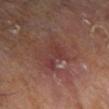follow-up — catalogued during a skin exam; not biopsied | imaging modality — 15 mm crop, total-body photography | subject — female, roughly 75 years of age | lesion size — about 4.5 mm | TBP lesion metrics — an eccentricity of roughly 0.85 and a shape-asymmetry score of about 0.45 (0 = symmetric); a lesion–skin lightness drop of about 6; border irregularity of about 5 on a 0–10 scale, a within-lesion color-variation index near 2.5/10, and radial color variation of about 1; a classifier nevus-likeness of about 0/100 and a detector confidence of about 80 out of 100 that the crop contains a lesion | body site — the left lower leg.